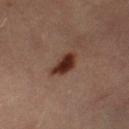<lesion>
  <biopsy_status>not biopsied; imaged during a skin examination</biopsy_status>
  <image>
    <source>total-body photography crop</source>
    <field_of_view_mm>15</field_of_view_mm>
  </image>
  <site>right lower leg</site>
  <automated_metrics>
    <border_irregularity_0_10>2.5</border_irregularity_0_10>
    <color_variation_0_10>3.0</color_variation_0_10>
    <peripheral_color_asymmetry>1.0</peripheral_color_asymmetry>
  </automated_metrics>
  <patient>
    <sex>female</sex>
    <age_approx>45</age_approx>
  </patient>
  <lesion_size>
    <long_diameter_mm_approx>3.5</long_diameter_mm_approx>
  </lesion_size>
  <lighting>cross-polarized</lighting>
</lesion>